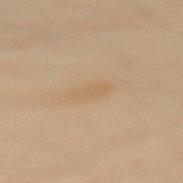notes: total-body-photography surveillance lesion; no biopsy | site: the mid back | TBP lesion metrics: a footprint of about 3.5 mm² and a symmetry-axis asymmetry near 0.3; a border-irregularity index near 3/10, a color-variation rating of about 0.5/10, and a peripheral color-asymmetry measure near 0 | illumination: cross-polarized | diameter: ~3 mm (longest diameter) | patient: female, in their 60s | image source: 15 mm crop, total-body photography.